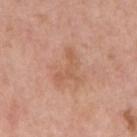Recorded during total-body skin imaging; not selected for excision or biopsy.
The lesion's longest dimension is about 4.5 mm.
Imaged with white-light lighting.
A female subject aged approximately 60.
The lesion is located on the right upper arm.
A 15 mm close-up extracted from a 3D total-body photography capture.